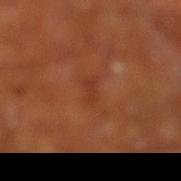Q: Was this lesion biopsied?
A: catalogued during a skin exam; not biopsied
Q: Who is the patient?
A: male, approximately 70 years of age
Q: Where on the body is the lesion?
A: the left lower leg
Q: How was this image acquired?
A: ~15 mm crop, total-body skin-cancer survey